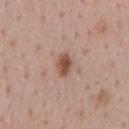Recorded during total-body skin imaging; not selected for excision or biopsy.
A female patient, aged around 45.
The tile uses white-light illumination.
The lesion's longest dimension is about 2.5 mm.
The lesion is located on the abdomen.
Cropped from a total-body skin-imaging series; the visible field is about 15 mm.
An algorithmic analysis of the crop reported a border-irregularity rating of about 1.5/10, internal color variation of about 2.5 on a 0–10 scale, and peripheral color asymmetry of about 1. The software also gave a nevus-likeness score of about 95/100 and a lesion-detection confidence of about 100/100.